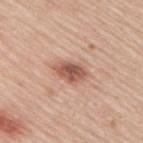Clinical impression: The lesion was tiled from a total-body skin photograph and was not biopsied. Acquisition and patient details: From the upper back. A 15 mm crop from a total-body photograph taken for skin-cancer surveillance. The patient is a male in their mid- to late 40s.